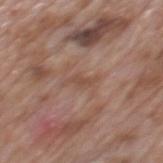| field | value |
|---|---|
| workup | no biopsy performed (imaged during a skin exam) |
| illumination | white-light |
| lesion size | about 3 mm |
| patient | male, aged 68–72 |
| imaging modality | 15 mm crop, total-body photography |
| body site | the mid back |